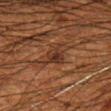<tbp_lesion>
<site>arm</site>
<patient>
  <sex>male</sex>
  <age_approx>50</age_approx>
</patient>
<image>
  <source>total-body photography crop</source>
  <field_of_view_mm>15</field_of_view_mm>
</image>
</tbp_lesion>